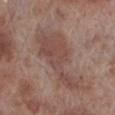Part of a total-body skin-imaging series; this lesion was reviewed on a skin check and was not flagged for biopsy. The patient is a male roughly 70 years of age. From the leg. The lesion's longest dimension is about 9 mm. The tile uses white-light illumination. Cropped from a total-body skin-imaging series; the visible field is about 15 mm.